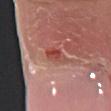Recorded during total-body skin imaging; not selected for excision or biopsy. Imaged with white-light lighting. A region of skin cropped from a whole-body photographic capture, roughly 15 mm wide. The lesion's longest dimension is about 3.5 mm. A female patient in their mid-60s. Located on the left forearm.